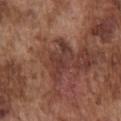  biopsy_status: not biopsied; imaged during a skin examination
  lesion_size:
    long_diameter_mm_approx: 5.0
  image:
    source: total-body photography crop
    field_of_view_mm: 15
  patient:
    sex: male
    age_approx: 75
  site: chest
  automated_metrics:
    cielab_L: 37
    cielab_a: 22
    cielab_b: 24
    vs_skin_darker_L: 8.0
    vs_skin_contrast_norm: 7.5